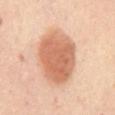Clinical impression:
This lesion was catalogued during total-body skin photography and was not selected for biopsy.
Acquisition and patient details:
About 7 mm across. A roughly 15 mm field-of-view crop from a total-body skin photograph. An algorithmic analysis of the crop reported a lesion area of about 27 mm², a shape eccentricity near 0.7, and a shape-asymmetry score of about 0.15 (0 = symmetric). It also reported a lesion color around L≈63 a*≈24 b*≈34 in CIELAB, about 14 CIELAB-L* units darker than the surrounding skin, and a normalized border contrast of about 8.5. The analysis additionally found internal color variation of about 4 on a 0–10 scale. The lesion is on the abdomen. The subject is a female about 45 years old.